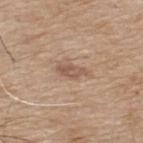Clinical impression:
Imaged during a routine full-body skin examination; the lesion was not biopsied and no histopathology is available.
Acquisition and patient details:
This is a white-light tile. Automated tile analysis of the lesion measured a border-irregularity rating of about 3/10, internal color variation of about 2 on a 0–10 scale, and radial color variation of about 0.5. The lesion's longest dimension is about 3.5 mm. The patient is a male in their mid-70s. From the back. A 15 mm close-up tile from a total-body photography series done for melanoma screening.